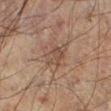Notes:
* size: about 4.5 mm
* location: the left leg
* image source: 15 mm crop, total-body photography
* automated metrics: a lesion area of about 12 mm², an outline eccentricity of about 0.5 (0 = round, 1 = elongated), and a shape-asymmetry score of about 0.35 (0 = symmetric); a nevus-likeness score of about 0/100
* subject: male, in their 60s